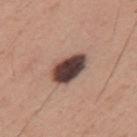Case summary:
* workup: total-body-photography surveillance lesion; no biopsy
* patient: male, approximately 30 years of age
* illumination: white-light illumination
* imaging modality: 15 mm crop, total-body photography
* location: the back
* lesion diameter: about 4.5 mm